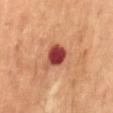{
  "biopsy_status": "not biopsied; imaged during a skin examination",
  "site": "abdomen",
  "patient": {
    "sex": "male",
    "age_approx": 60
  },
  "automated_metrics": {
    "area_mm2_approx": 7.0,
    "eccentricity": 0.6,
    "cielab_L": 39,
    "cielab_a": 30,
    "cielab_b": 26,
    "vs_skin_darker_L": 17.0,
    "vs_skin_contrast_norm": 13.5,
    "nevus_likeness_0_100": 5,
    "lesion_detection_confidence_0_100": 100
  },
  "image": {
    "source": "total-body photography crop",
    "field_of_view_mm": 15
  }
}